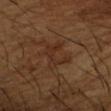Part of a total-body skin-imaging series; this lesion was reviewed on a skin check and was not flagged for biopsy. This is a cross-polarized tile. The subject is a male roughly 65 years of age. A lesion tile, about 15 mm wide, cut from a 3D total-body photograph. The lesion is located on the right forearm. The lesion's longest dimension is about 4 mm.